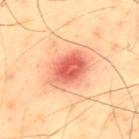Q: Is there a histopathology result?
A: catalogued during a skin exam; not biopsied